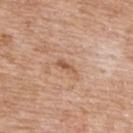{"biopsy_status": "not biopsied; imaged during a skin examination", "lighting": "white-light", "lesion_size": {"long_diameter_mm_approx": 2.5}, "site": "upper back", "image": {"source": "total-body photography crop", "field_of_view_mm": 15}, "patient": {"sex": "male", "age_approx": 65}}